An algorithmic analysis of the crop reported an outline eccentricity of about 0.55 (0 = round, 1 = elongated) and two-axis asymmetry of about 0.15. And it measured a mean CIELAB color near L≈55 a*≈25 b*≈29, about 11 CIELAB-L* units darker than the surrounding skin, and a normalized lesion–skin contrast near 7.5. And it measured a classifier nevus-likeness of about 20/100 and a detector confidence of about 100 out of 100 that the crop contains a lesion. A 15 mm close-up extracted from a 3D total-body photography capture. The lesion is located on the right upper arm. The lesion's longest dimension is about 6 mm. A male patient, aged 18 to 22 — 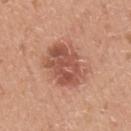Notes:
• histopathology · a dysplastic (Clark) nevus — a benign lesion